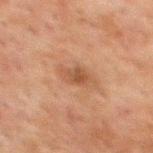Clinical impression:
No biopsy was performed on this lesion — it was imaged during a full skin examination and was not determined to be concerning.
Acquisition and patient details:
The lesion is located on the mid back. Captured under cross-polarized illumination. A male subject roughly 60 years of age. The lesion-visualizer software estimated an automated nevus-likeness rating near 10 out of 100. Approximately 3 mm at its widest. A 15 mm close-up extracted from a 3D total-body photography capture.